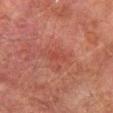{"biopsy_status": "not biopsied; imaged during a skin examination", "site": "left thigh", "patient": {"sex": "male", "age_approx": 75}, "automated_metrics": {"area_mm2_approx": 3.0, "eccentricity": 0.9, "shape_asymmetry": 0.25, "cielab_L": 39, "cielab_a": 26, "cielab_b": 26, "vs_skin_darker_L": 5.0, "vs_skin_contrast_norm": 4.5, "lesion_detection_confidence_0_100": 100}, "image": {"source": "total-body photography crop", "field_of_view_mm": 15}, "lighting": "cross-polarized"}